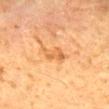Image and clinical context: Longest diameter approximately 3.5 mm. A roughly 15 mm field-of-view crop from a total-body skin photograph. A male patient about 60 years old. An algorithmic analysis of the crop reported an average lesion color of about L≈55 a*≈22 b*≈40 (CIELAB) and a lesion-to-skin contrast of about 6 (normalized; higher = more distinct). And it measured a border-irregularity index near 3.5/10, a within-lesion color-variation index near 2.5/10, and a peripheral color-asymmetry measure near 1. It also reported a classifier nevus-likeness of about 0/100. On the mid back. This is a cross-polarized tile.